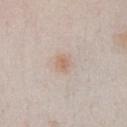Assessment:
Recorded during total-body skin imaging; not selected for excision or biopsy.
Clinical summary:
Located on the chest. A female patient, roughly 40 years of age. Captured under white-light illumination. The lesion's longest dimension is about 2.5 mm. Cropped from a total-body skin-imaging series; the visible field is about 15 mm.